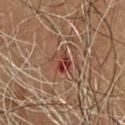Part of a total-body skin-imaging series; this lesion was reviewed on a skin check and was not flagged for biopsy. A close-up tile cropped from a whole-body skin photograph, about 15 mm across. A male subject approximately 65 years of age. From the chest.